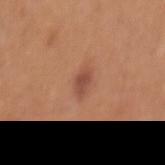{"biopsy_status": "not biopsied; imaged during a skin examination", "site": "back", "patient": {"sex": "male", "age_approx": 55}, "lighting": "white-light", "image": {"source": "total-body photography crop", "field_of_view_mm": 15}}